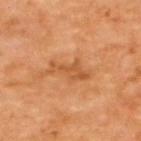{
  "biopsy_status": "not biopsied; imaged during a skin examination",
  "patient": {
    "age_approx": 65
  },
  "image": {
    "source": "total-body photography crop",
    "field_of_view_mm": 15
  },
  "site": "back",
  "lesion_size": {
    "long_diameter_mm_approx": 5.0
  },
  "lighting": "cross-polarized"
}